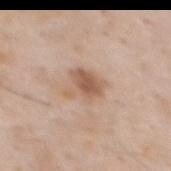The lesion was photographed on a routine skin check and not biopsied; there is no pathology result. A roughly 15 mm field-of-view crop from a total-body skin photograph. Captured under white-light illumination. The recorded lesion diameter is about 3.5 mm. The patient is a male approximately 60 years of age. The lesion is on the mid back.